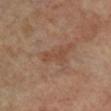Imaged during a routine full-body skin examination; the lesion was not biopsied and no histopathology is available. From the left leg. The patient is a female aged 63 to 67. A 15 mm crop from a total-body photograph taken for skin-cancer surveillance.